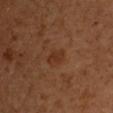Case summary:
– biopsy status: imaged on a skin check; not biopsied
– image: 15 mm crop, total-body photography
– automated lesion analysis: a mean CIELAB color near L≈32 a*≈22 b*≈31, about 6 CIELAB-L* units darker than the surrounding skin, and a normalized border contrast of about 6.5; a border-irregularity index near 3.5/10, internal color variation of about 0.5 on a 0–10 scale, and radial color variation of about 0
– illumination: cross-polarized
– patient: male, approximately 50 years of age
– lesion size: ~2.5 mm (longest diameter)
– site: the front of the torso